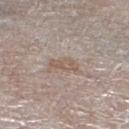Impression: The lesion was photographed on a routine skin check and not biopsied; there is no pathology result. Clinical summary: Cropped from a whole-body photographic skin survey; the tile spans about 15 mm. A male patient, aged approximately 80. The tile uses white-light illumination. Automated image analysis of the tile measured a lesion area of about 4 mm². It also reported an average lesion color of about L≈56 a*≈14 b*≈25 (CIELAB), roughly 7 lightness units darker than nearby skin, and a normalized lesion–skin contrast near 6. And it measured a nevus-likeness score of about 0/100 and lesion-presence confidence of about 60/100. From the left lower leg.